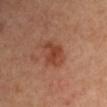This lesion was catalogued during total-body skin photography and was not selected for biopsy. A female patient, aged 43 to 47. About 4 mm across. A region of skin cropped from a whole-body photographic capture, roughly 15 mm wide. The lesion is located on the left upper arm.